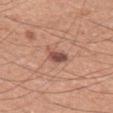This lesion was catalogued during total-body skin photography and was not selected for biopsy.
A male patient aged 53 to 57.
Located on the leg.
The recorded lesion diameter is about 3 mm.
The tile uses white-light illumination.
The total-body-photography lesion software estimated a mean CIELAB color near L≈51 a*≈23 b*≈26, about 12 CIELAB-L* units darker than the surrounding skin, and a normalized lesion–skin contrast near 9. The software also gave a border-irregularity rating of about 3/10, a within-lesion color-variation index near 3.5/10, and peripheral color asymmetry of about 1. The software also gave a classifier nevus-likeness of about 80/100 and lesion-presence confidence of about 100/100.
Cropped from a total-body skin-imaging series; the visible field is about 15 mm.